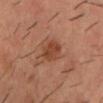Recorded during total-body skin imaging; not selected for excision or biopsy. The lesion-visualizer software estimated a footprint of about 6 mm², an eccentricity of roughly 0.8, and two-axis asymmetry of about 0.2. And it measured a lesion color around L≈42 a*≈25 b*≈31 in CIELAB, about 9 CIELAB-L* units darker than the surrounding skin, and a normalized border contrast of about 7.5. The software also gave a nevus-likeness score of about 80/100 and a lesion-detection confidence of about 100/100. The lesion's longest dimension is about 3.5 mm. A close-up tile cropped from a whole-body skin photograph, about 15 mm across. The lesion is on the front of the torso. The patient is a male aged 38 to 42.